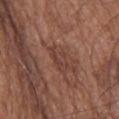The lesion was tiled from a total-body skin photograph and was not biopsied. A lesion tile, about 15 mm wide, cut from a 3D total-body photograph. The patient is a male approximately 75 years of age. The tile uses white-light illumination. On the upper back. The lesion-visualizer software estimated a footprint of about 5 mm², a shape eccentricity near 0.85, and a shape-asymmetry score of about 0.25 (0 = symmetric). The analysis additionally found a lesion color around L≈40 a*≈21 b*≈25 in CIELAB, a lesion–skin lightness drop of about 6, and a lesion-to-skin contrast of about 5.5 (normalized; higher = more distinct). And it measured a border-irregularity index near 3.5/10 and radial color variation of about 1.